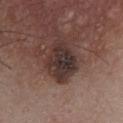A male subject aged approximately 55.
The lesion is on the front of the torso.
Approximately 5.5 mm at its widest.
Imaged with white-light lighting.
A region of skin cropped from a whole-body photographic capture, roughly 15 mm wide.